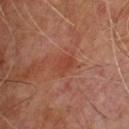Imaged during a routine full-body skin examination; the lesion was not biopsied and no histopathology is available. A 15 mm close-up tile from a total-body photography series done for melanoma screening. Longest diameter approximately 2.5 mm. A male subject, in their mid- to late 60s. The tile uses cross-polarized illumination. Automated image analysis of the tile measured a footprint of about 3.5 mm², a shape eccentricity near 0.7, and a shape-asymmetry score of about 0.2 (0 = symmetric). The software also gave a mean CIELAB color near L≈42 a*≈28 b*≈30, a lesion–skin lightness drop of about 6, and a normalized border contrast of about 5. The analysis additionally found border irregularity of about 1.5 on a 0–10 scale, internal color variation of about 2 on a 0–10 scale, and peripheral color asymmetry of about 0.5.